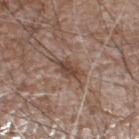Case summary:
– follow-up: no biopsy performed (imaged during a skin exam)
– automated lesion analysis: an area of roughly 4.5 mm², an outline eccentricity of about 0.8 (0 = round, 1 = elongated), and a symmetry-axis asymmetry near 0.3; an average lesion color of about L≈44 a*≈17 b*≈25 (CIELAB), a lesion–skin lightness drop of about 10, and a lesion-to-skin contrast of about 8 (normalized; higher = more distinct); a border-irregularity index near 3/10, internal color variation of about 2.5 on a 0–10 scale, and a peripheral color-asymmetry measure near 0.5; lesion-presence confidence of about 100/100
– imaging modality: ~15 mm tile from a whole-body skin photo
– tile lighting: white-light illumination
– patient: male, about 60 years old
– location: the leg